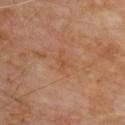{"biopsy_status": "not biopsied; imaged during a skin examination", "patient": {"sex": "male", "age_approx": 70}, "lesion_size": {"long_diameter_mm_approx": 2.5}, "site": "back", "image": {"source": "total-body photography crop", "field_of_view_mm": 15}}